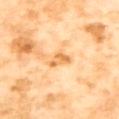Assessment:
No biopsy was performed on this lesion — it was imaged during a full skin examination and was not determined to be concerning.
Background:
A female patient about 55 years old. Located on the back. This image is a 15 mm lesion crop taken from a total-body photograph.